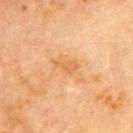Assessment: Part of a total-body skin-imaging series; this lesion was reviewed on a skin check and was not flagged for biopsy. Image and clinical context: The patient is a male roughly 70 years of age. The lesion's longest dimension is about 3 mm. Located on the front of the torso. The tile uses cross-polarized illumination. A 15 mm close-up extracted from a 3D total-body photography capture.